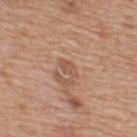Imaged during a routine full-body skin examination; the lesion was not biopsied and no histopathology is available.
A 15 mm close-up extracted from a 3D total-body photography capture.
The lesion is on the upper back.
A male subject about 60 years old.